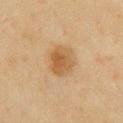Imaged during a routine full-body skin examination; the lesion was not biopsied and no histopathology is available. On the chest. A female patient, aged 53 to 57. A region of skin cropped from a whole-body photographic capture, roughly 15 mm wide.